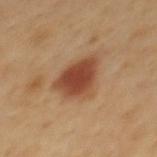follow-up = imaged on a skin check; not biopsied
tile lighting = cross-polarized illumination
patient = male, aged 48 to 52
image = 15 mm crop, total-body photography
diameter = ≈4.5 mm
body site = the mid back